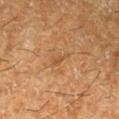Impression:
This lesion was catalogued during total-body skin photography and was not selected for biopsy.
Background:
A male patient in their 60s. Approximately 2.5 mm at its widest. A region of skin cropped from a whole-body photographic capture, roughly 15 mm wide. Captured under cross-polarized illumination. The lesion is located on the left lower leg. The total-body-photography lesion software estimated a lesion area of about 2.5 mm², a shape eccentricity near 0.9, and two-axis asymmetry of about 0.5. The software also gave a lesion–skin lightness drop of about 7 and a lesion-to-skin contrast of about 5 (normalized; higher = more distinct). And it measured a border-irregularity index near 5.5/10, a color-variation rating of about 0/10, and peripheral color asymmetry of about 0. The software also gave an automated nevus-likeness rating near 0 out of 100 and lesion-presence confidence of about 95/100.